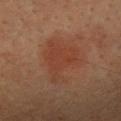  site: back
  patient:
    sex: male
    age_approx: 40
  image:
    source: total-body photography crop
    field_of_view_mm: 15